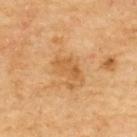Q: How was this image acquired?
A: total-body-photography crop, ~15 mm field of view
Q: What are the patient's age and sex?
A: male, about 70 years old
Q: Lesion location?
A: the upper back